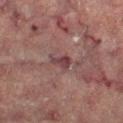Findings:
– notes: imaged on a skin check; not biopsied
– automated metrics: a nevus-likeness score of about 0/100 and a detector confidence of about 75 out of 100 that the crop contains a lesion
– image source: total-body-photography crop, ~15 mm field of view
– lighting: cross-polarized illumination
– subject: female, aged around 70
– location: the right lower leg